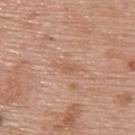Imaged during a routine full-body skin examination; the lesion was not biopsied and no histopathology is available. The lesion is located on the upper back. The recorded lesion diameter is about 3 mm. A female patient, roughly 60 years of age. Cropped from a whole-body photographic skin survey; the tile spans about 15 mm.